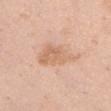  biopsy_status: not biopsied; imaged during a skin examination
  site: chest
  patient:
    sex: female
    age_approx: 65
  image:
    source: total-body photography crop
    field_of_view_mm: 15
  lighting: white-light